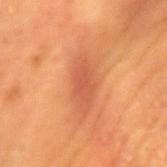| key | value |
|---|---|
| workup | total-body-photography surveillance lesion; no biopsy |
| patient | male, approximately 60 years of age |
| body site | the left upper arm |
| TBP lesion metrics | a footprint of about 5.5 mm² and an eccentricity of roughly 0.85 |
| diameter | ≈3.5 mm |
| tile lighting | cross-polarized |
| image source | total-body-photography crop, ~15 mm field of view |